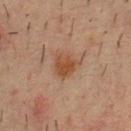Impression: The lesion was tiled from a total-body skin photograph and was not biopsied. Acquisition and patient details: Located on the chest. Captured under cross-polarized illumination. A close-up tile cropped from a whole-body skin photograph, about 15 mm across. A male subject aged approximately 35.